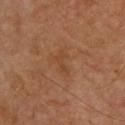Captured during whole-body skin photography for melanoma surveillance; the lesion was not biopsied. This is a cross-polarized tile. A close-up tile cropped from a whole-body skin photograph, about 15 mm across. Approximately 3 mm at its widest. The lesion is located on the upper back. Automated image analysis of the tile measured roughly 5 lightness units darker than nearby skin and a normalized lesion–skin contrast near 5. A male subject, roughly 60 years of age.